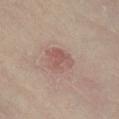<tbp_lesion>
  <biopsy_status>not biopsied; imaged during a skin examination</biopsy_status>
  <site>leg</site>
  <image>
    <source>total-body photography crop</source>
    <field_of_view_mm>15</field_of_view_mm>
  </image>
  <patient>
    <sex>female</sex>
    <age_approx>35</age_approx>
  </patient>
</tbp_lesion>